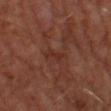Impression: This lesion was catalogued during total-body skin photography and was not selected for biopsy. Background: From the leg. A region of skin cropped from a whole-body photographic capture, roughly 15 mm wide. The subject is a male aged 58–62. The recorded lesion diameter is about 3.5 mm. Imaged with cross-polarized lighting.